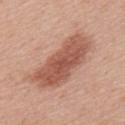Q: Where on the body is the lesion?
A: the upper back
Q: Patient demographics?
A: male, aged approximately 65
Q: What is the lesion's diameter?
A: ≈8 mm
Q: Illumination type?
A: white-light illumination
Q: What is the imaging modality?
A: total-body-photography crop, ~15 mm field of view
Q: What did automated image analysis measure?
A: a lesion area of about 25 mm², an outline eccentricity of about 0.9 (0 = round, 1 = elongated), and two-axis asymmetry of about 0.2; a classifier nevus-likeness of about 30/100 and a detector confidence of about 100 out of 100 that the crop contains a lesion